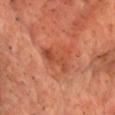The lesion was tiled from a total-body skin photograph and was not biopsied.
From the chest.
A male subject, aged approximately 65.
The total-body-photography lesion software estimated roughly 8 lightness units darker than nearby skin and a normalized lesion–skin contrast near 6. The software also gave border irregularity of about 5 on a 0–10 scale and a color-variation rating of about 5/10.
This is a cross-polarized tile.
This image is a 15 mm lesion crop taken from a total-body photograph.
Longest diameter approximately 5 mm.